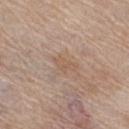Q: Was a biopsy performed?
A: no biopsy performed (imaged during a skin exam)
Q: What is the lesion's diameter?
A: ≈3 mm
Q: Who is the patient?
A: female, in their mid-60s
Q: What lighting was used for the tile?
A: white-light illumination
Q: Lesion location?
A: the left leg
Q: How was this image acquired?
A: 15 mm crop, total-body photography